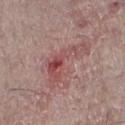workup = no biopsy performed (imaged during a skin exam) | imaging modality = 15 mm crop, total-body photography | body site = the left lower leg | subject = male, aged approximately 55.